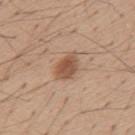Findings:
- notes · catalogued during a skin exam; not biopsied
- body site · the back
- patient · male, aged 53–57
- image source · 15 mm crop, total-body photography